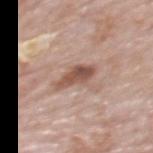Imaged with white-light lighting.
A 15 mm crop from a total-body photograph taken for skin-cancer surveillance.
A male subject about 80 years old.
On the mid back.
The recorded lesion diameter is about 4 mm.